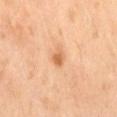Imaged during a routine full-body skin examination; the lesion was not biopsied and no histopathology is available.
A lesion tile, about 15 mm wide, cut from a 3D total-body photograph.
This is a cross-polarized tile.
A male subject aged approximately 70.
Located on the leg.